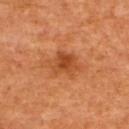workup: catalogued during a skin exam; not biopsied
body site: the upper back
patient: male, aged 58 to 62
image: total-body-photography crop, ~15 mm field of view